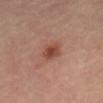biopsy status = imaged on a skin check; not biopsied
site = the mid back
image = 15 mm crop, total-body photography
image-analysis metrics = a shape eccentricity near 0.5 and two-axis asymmetry of about 0.2; a border-irregularity rating of about 2/10, a color-variation rating of about 3.5/10, and a peripheral color-asymmetry measure near 1; a nevus-likeness score of about 75/100 and lesion-presence confidence of about 100/100
patient = male, in their mid- to late 50s
lesion size = ≈2.5 mm
illumination = cross-polarized illumination